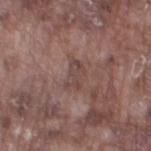Case summary:
* size: ≈3 mm
* patient: male, aged around 75
* image-analysis metrics: an average lesion color of about L≈44 a*≈18 b*≈20 (CIELAB) and roughly 6 lightness units darker than nearby skin; a border-irregularity index near 3/10, internal color variation of about 2 on a 0–10 scale, and peripheral color asymmetry of about 0.5; a classifier nevus-likeness of about 0/100 and a lesion-detection confidence of about 95/100
* imaging modality: total-body-photography crop, ~15 mm field of view
* anatomic site: the left thigh